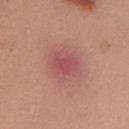Part of a total-body skin-imaging series; this lesion was reviewed on a skin check and was not flagged for biopsy.
A female subject in their mid- to late 20s.
The total-body-photography lesion software estimated an area of roughly 6.5 mm². And it measured a mean CIELAB color near L≈51 a*≈31 b*≈22, roughly 8 lightness units darker than nearby skin, and a normalized lesion–skin contrast near 6. It also reported border irregularity of about 2.5 on a 0–10 scale, a within-lesion color-variation index near 2/10, and a peripheral color-asymmetry measure near 0.5. It also reported a classifier nevus-likeness of about 0/100.
The recorded lesion diameter is about 3.5 mm.
A lesion tile, about 15 mm wide, cut from a 3D total-body photograph.
This is a white-light tile.
On the mid back.